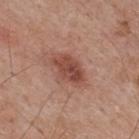Q: Was a biopsy performed?
A: no biopsy performed (imaged during a skin exam)
Q: Who is the patient?
A: male, roughly 70 years of age
Q: What kind of image is this?
A: ~15 mm crop, total-body skin-cancer survey
Q: What is the anatomic site?
A: the upper back
Q: What is the lesion's diameter?
A: ≈4.5 mm